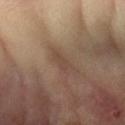The lesion was photographed on a routine skin check and not biopsied; there is no pathology result. Cropped from a total-body skin-imaging series; the visible field is about 15 mm. The lesion is on the arm. A female subject in their mid- to late 70s.